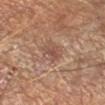Part of a total-body skin-imaging series; this lesion was reviewed on a skin check and was not flagged for biopsy. A 15 mm close-up tile from a total-body photography series done for melanoma screening. Automated tile analysis of the lesion measured a shape eccentricity near 0.85 and a shape-asymmetry score of about 0.4 (0 = symmetric). The analysis additionally found a mean CIELAB color near L≈50 a*≈21 b*≈27, about 9 CIELAB-L* units darker than the surrounding skin, and a normalized lesion–skin contrast near 6.5. The analysis additionally found a within-lesion color-variation index near 1/10 and a peripheral color-asymmetry measure near 0. Located on the left forearm. The recorded lesion diameter is about 3 mm. Captured under cross-polarized illumination.